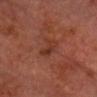Impression: This lesion was catalogued during total-body skin photography and was not selected for biopsy. Context: The subject is a male approximately 75 years of age. From the chest. A lesion tile, about 15 mm wide, cut from a 3D total-body photograph. The tile uses cross-polarized illumination.